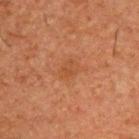| feature | finding |
|---|---|
| lighting | cross-polarized |
| imaging modality | ~15 mm tile from a whole-body skin photo |
| lesion diameter | ≈2.5 mm |
| patient | male, aged 48–52 |
| location | the upper back |